A male subject, approximately 55 years of age; this is a white-light tile; cropped from a whole-body photographic skin survey; the tile spans about 15 mm; the lesion's longest dimension is about 2 mm; the lesion is located on the head or neck; The lesion-visualizer software estimated a shape eccentricity near 0.75 and a shape-asymmetry score of about 0.3 (0 = symmetric). And it measured a border-irregularity rating of about 3/10 and a peripheral color-asymmetry measure near 0.
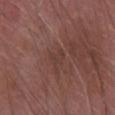– biopsy diagnosis · a squamous cell carcinoma in situ — a malignant lesion